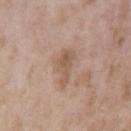Clinical impression: The lesion was photographed on a routine skin check and not biopsied; there is no pathology result. Acquisition and patient details: The lesion is on the left upper arm. Approximately 4.5 mm at its widest. A male patient, aged around 60. Imaged with white-light lighting. A region of skin cropped from a whole-body photographic capture, roughly 15 mm wide.